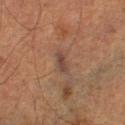Q: Was a biopsy performed?
A: total-body-photography surveillance lesion; no biopsy
Q: Automated lesion metrics?
A: an area of roughly 4 mm², a shape eccentricity near 0.85, and a symmetry-axis asymmetry near 0.25; a mean CIELAB color near L≈34 a*≈15 b*≈20, a lesion–skin lightness drop of about 6, and a normalized border contrast of about 7.5; an automated nevus-likeness rating near 0 out of 100 and lesion-presence confidence of about 90/100
Q: Lesion size?
A: ≈3 mm
Q: What is the imaging modality?
A: ~15 mm tile from a whole-body skin photo
Q: What are the patient's age and sex?
A: male, about 75 years old
Q: Lesion location?
A: the left lower leg
Q: How was the tile lit?
A: cross-polarized illumination